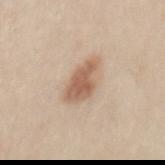Impression:
The lesion was photographed on a routine skin check and not biopsied; there is no pathology result.
Background:
A female subject aged approximately 40. The lesion is located on the back. Captured under white-light illumination. The total-body-photography lesion software estimated a lesion area of about 9.5 mm², a shape eccentricity near 0.85, and a shape-asymmetry score of about 0.25 (0 = symmetric). It also reported an average lesion color of about L≈60 a*≈18 b*≈30 (CIELAB), roughly 12 lightness units darker than nearby skin, and a normalized border contrast of about 8. And it measured a color-variation rating of about 3/10 and peripheral color asymmetry of about 1. The software also gave a nevus-likeness score of about 95/100. Cropped from a total-body skin-imaging series; the visible field is about 15 mm. Measured at roughly 5 mm in maximum diameter.